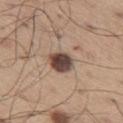{
  "site": "left thigh",
  "image": {
    "source": "total-body photography crop",
    "field_of_view_mm": 15
  },
  "patient": {
    "sex": "male",
    "age_approx": 65
  }
}